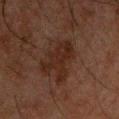Context: Imaged with cross-polarized lighting. Automated image analysis of the tile measured roughly 6 lightness units darker than nearby skin. It also reported border irregularity of about 6.5 on a 0–10 scale, a color-variation rating of about 2.5/10, and radial color variation of about 1. The lesion's longest dimension is about 5 mm. From the chest. A male subject, aged approximately 50. A close-up tile cropped from a whole-body skin photograph, about 15 mm across.